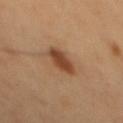Imaged during a routine full-body skin examination; the lesion was not biopsied and no histopathology is available.
Approximately 3.5 mm at its widest.
A male subject aged approximately 50.
The lesion-visualizer software estimated a lesion area of about 6.5 mm², an eccentricity of roughly 0.8, and a shape-asymmetry score of about 0.15 (0 = symmetric). It also reported an average lesion color of about L≈44 a*≈21 b*≈33 (CIELAB) and roughly 12 lightness units darker than nearby skin. And it measured internal color variation of about 2 on a 0–10 scale and peripheral color asymmetry of about 0.5. It also reported a detector confidence of about 100 out of 100 that the crop contains a lesion.
The tile uses cross-polarized illumination.
A 15 mm close-up tile from a total-body photography series done for melanoma screening.
From the back.